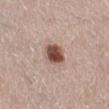Part of a total-body skin-imaging series; this lesion was reviewed on a skin check and was not flagged for biopsy.
The patient is a female aged 38 to 42.
Longest diameter approximately 3 mm.
A region of skin cropped from a whole-body photographic capture, roughly 15 mm wide.
The total-body-photography lesion software estimated a lesion–skin lightness drop of about 17 and a lesion-to-skin contrast of about 11.5 (normalized; higher = more distinct). It also reported an automated nevus-likeness rating near 100 out of 100 and lesion-presence confidence of about 100/100.
The lesion is located on the right lower leg.